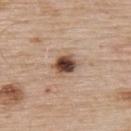body site = the upper back | subject = male, about 55 years old | lighting = white-light illumination | size = about 3 mm | acquisition = ~15 mm crop, total-body skin-cancer survey.